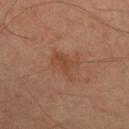Notes:
– biopsy status — imaged on a skin check; not biopsied
– anatomic site — the right lower leg
– image — ~15 mm tile from a whole-body skin photo
– patient — male, aged approximately 65